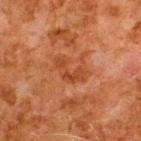biopsy_status: not biopsied; imaged during a skin examination
lesion_size:
  long_diameter_mm_approx: 4.5
lighting: cross-polarized
patient:
  sex: male
  age_approx: 80
automated_metrics:
  cielab_L: 36
  cielab_a: 24
  cielab_b: 32
  vs_skin_contrast_norm: 6.0
  peripheral_color_asymmetry: 0.5
  nevus_likeness_0_100: 0
  lesion_detection_confidence_0_100: 100
site: upper back
image:
  source: total-body photography crop
  field_of_view_mm: 15